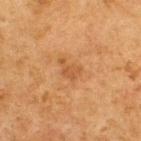No biopsy was performed on this lesion — it was imaged during a full skin examination and was not determined to be concerning. Imaged with cross-polarized lighting. A roughly 15 mm field-of-view crop from a total-body skin photograph. On the upper back. Approximately 3 mm at its widest. The patient is a male roughly 75 years of age.